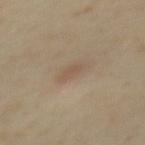Findings:
• subject · male, aged approximately 55
• tile lighting · cross-polarized illumination
• lesion size · ~3 mm (longest diameter)
• image · total-body-photography crop, ~15 mm field of view
• anatomic site · the mid back